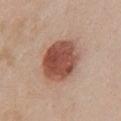Impression: This lesion was catalogued during total-body skin photography and was not selected for biopsy. Clinical summary: A roughly 15 mm field-of-view crop from a total-body skin photograph. The lesion is located on the chest. The recorded lesion diameter is about 5.5 mm. This is a white-light tile. A female patient in their 40s.